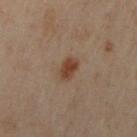biopsy status = no biopsy performed (imaged during a skin exam)
subject = male, in their 50s
site = the left upper arm
imaging modality = ~15 mm crop, total-body skin-cancer survey
size = ≈2.5 mm